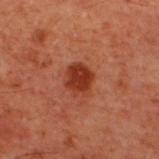{
  "biopsy_status": "not biopsied; imaged during a skin examination",
  "automated_metrics": {
    "color_variation_0_10": 2.5,
    "peripheral_color_asymmetry": 1.0,
    "nevus_likeness_0_100": 95,
    "lesion_detection_confidence_0_100": 100
  },
  "site": "back",
  "patient": {
    "sex": "male",
    "age_approx": 60
  },
  "lesion_size": {
    "long_diameter_mm_approx": 3.5
  },
  "image": {
    "source": "total-body photography crop",
    "field_of_view_mm": 15
  }
}